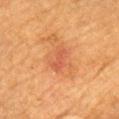Part of a total-body skin-imaging series; this lesion was reviewed on a skin check and was not flagged for biopsy.
The tile uses cross-polarized illumination.
The subject is a male aged 83 or over.
Cropped from a total-body skin-imaging series; the visible field is about 15 mm.
Located on the head or neck.